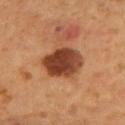Findings:
• workup · imaged on a skin check; not biopsied
• patient · male, aged approximately 55
• image · 15 mm crop, total-body photography
• tile lighting · cross-polarized illumination
• location · the mid back
• TBP lesion metrics · a footprint of about 15 mm², an outline eccentricity of about 0.65 (0 = round, 1 = elongated), and two-axis asymmetry of about 0.15; a border-irregularity index near 1/10, internal color variation of about 5 on a 0–10 scale, and radial color variation of about 1.5; an automated nevus-likeness rating near 90 out of 100 and a detector confidence of about 100 out of 100 that the crop contains a lesion